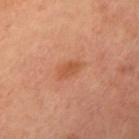  biopsy_status: not biopsied; imaged during a skin examination